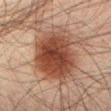A 15 mm crop from a total-body photograph taken for skin-cancer surveillance. On the lower back. Longest diameter approximately 7 mm. A male patient, aged around 70. Captured under cross-polarized illumination.